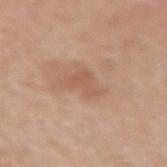Findings:
- follow-up — catalogued during a skin exam; not biopsied
- site — the left forearm
- lesion size — ≈3.5 mm
- subject — female, approximately 65 years of age
- image-analysis metrics — border irregularity of about 5.5 on a 0–10 scale, internal color variation of about 1 on a 0–10 scale, and peripheral color asymmetry of about 0.5; an automated nevus-likeness rating near 0 out of 100 and a detector confidence of about 100 out of 100 that the crop contains a lesion
- lighting — white-light illumination
- imaging modality — total-body-photography crop, ~15 mm field of view